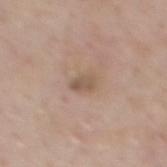| field | value |
|---|---|
| follow-up | catalogued during a skin exam; not biopsied |
| tile lighting | white-light |
| automated metrics | a border-irregularity index near 2.5/10, internal color variation of about 2.5 on a 0–10 scale, and radial color variation of about 0.5; a nevus-likeness score of about 0/100 and a detector confidence of about 100 out of 100 that the crop contains a lesion |
| lesion diameter | ~2.5 mm (longest diameter) |
| subject | male, about 55 years old |
| site | the back |
| acquisition | total-body-photography crop, ~15 mm field of view |